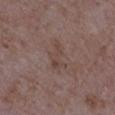{"biopsy_status": "not biopsied; imaged during a skin examination", "lighting": "white-light", "lesion_size": {"long_diameter_mm_approx": 3.5}, "patient": {"sex": "male", "age_approx": 65}, "site": "left upper arm", "image": {"source": "total-body photography crop", "field_of_view_mm": 15}}